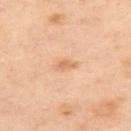Q: Was a biopsy performed?
A: no biopsy performed (imaged during a skin exam)
Q: Who is the patient?
A: female, in their 40s
Q: Illumination type?
A: cross-polarized illumination
Q: What is the imaging modality?
A: ~15 mm crop, total-body skin-cancer survey
Q: What is the anatomic site?
A: the upper back
Q: Automated lesion metrics?
A: an area of roughly 2.5 mm², an outline eccentricity of about 0.9 (0 = round, 1 = elongated), and two-axis asymmetry of about 0.35; a border-irregularity rating of about 3.5/10, a color-variation rating of about 0/10, and peripheral color asymmetry of about 0
Q: How large is the lesion?
A: ~2.5 mm (longest diameter)